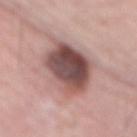Impression:
Captured during whole-body skin photography for melanoma surveillance; the lesion was not biopsied.
Acquisition and patient details:
This is a white-light tile. The subject is a male roughly 65 years of age. A 15 mm close-up extracted from a 3D total-body photography capture. From the mid back. About 9 mm across.